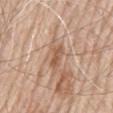Assessment: The lesion was photographed on a routine skin check and not biopsied; there is no pathology result. Acquisition and patient details: The lesion is located on the mid back. This is a white-light tile. About 3 mm across. The patient is a male approximately 80 years of age. An algorithmic analysis of the crop reported a footprint of about 3.5 mm², a shape eccentricity near 0.85, and a symmetry-axis asymmetry near 0.2. The software also gave a lesion color around L≈56 a*≈21 b*≈31 in CIELAB, about 9 CIELAB-L* units darker than the surrounding skin, and a normalized lesion–skin contrast near 6.5. It also reported a border-irregularity index near 2/10, internal color variation of about 2.5 on a 0–10 scale, and a peripheral color-asymmetry measure near 1. The analysis additionally found a detector confidence of about 95 out of 100 that the crop contains a lesion. A 15 mm crop from a total-body photograph taken for skin-cancer surveillance.